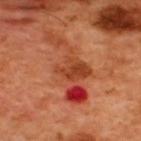| feature | finding |
|---|---|
| anatomic site | the back |
| illumination | cross-polarized |
| imaging modality | 15 mm crop, total-body photography |
| image-analysis metrics | a border-irregularity rating of about 9/10, a within-lesion color-variation index near 6.5/10, and radial color variation of about 1 |
| subject | male, about 50 years old |